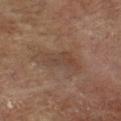Q: Is there a histopathology result?
A: catalogued during a skin exam; not biopsied
Q: How was the tile lit?
A: cross-polarized illumination
Q: What kind of image is this?
A: total-body-photography crop, ~15 mm field of view
Q: What is the anatomic site?
A: the chest
Q: What are the patient's age and sex?
A: female, aged around 80